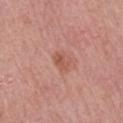<tbp_lesion>
<biopsy_status>not biopsied; imaged during a skin examination</biopsy_status>
<image>
  <source>total-body photography crop</source>
  <field_of_view_mm>15</field_of_view_mm>
</image>
<automated_metrics>
  <cielab_L>54</cielab_L>
  <cielab_a>26</cielab_a>
  <cielab_b>29</cielab_b>
  <vs_skin_darker_L>8.0</vs_skin_darker_L>
  <vs_skin_contrast_norm>6.0</vs_skin_contrast_norm>
  <border_irregularity_0_10>3.0</border_irregularity_0_10>
  <color_variation_0_10>3.0</color_variation_0_10>
  <nevus_likeness_0_100>15</nevus_likeness_0_100>
  <lesion_detection_confidence_0_100>100</lesion_detection_confidence_0_100>
</automated_metrics>
<patient>
  <sex>male</sex>
  <age_approx>75</age_approx>
</patient>
<site>right upper arm</site>
<lesion_size>
  <long_diameter_mm_approx>2.5</long_diameter_mm_approx>
</lesion_size>
</tbp_lesion>